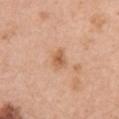The lesion was photographed on a routine skin check and not biopsied; there is no pathology result.
Captured under white-light illumination.
A lesion tile, about 15 mm wide, cut from a 3D total-body photograph.
On the right upper arm.
The lesion's longest dimension is about 2.5 mm.
A female patient approximately 60 years of age.